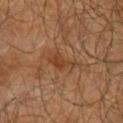Assessment: Part of a total-body skin-imaging series; this lesion was reviewed on a skin check and was not flagged for biopsy.